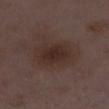No biopsy was performed on this lesion — it was imaged during a full skin examination and was not determined to be concerning. Cropped from a whole-body photographic skin survey; the tile spans about 15 mm. The tile uses white-light illumination. The lesion-visualizer software estimated a classifier nevus-likeness of about 70/100 and a detector confidence of about 100 out of 100 that the crop contains a lesion. Located on the right lower leg. The recorded lesion diameter is about 5 mm. A female patient in their 30s.